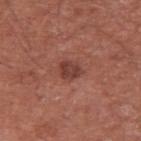Clinical impression:
Part of a total-body skin-imaging series; this lesion was reviewed on a skin check and was not flagged for biopsy.
Context:
The total-body-photography lesion software estimated a border-irregularity index near 2/10, a within-lesion color-variation index near 2/10, and peripheral color asymmetry of about 0.5. And it measured a nevus-likeness score of about 40/100. The lesion is on the arm. A male subject, aged approximately 65. Approximately 3 mm at its widest. Cropped from a whole-body photographic skin survey; the tile spans about 15 mm. This is a white-light tile.